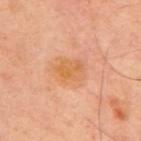Automated image analysis of the tile measured a footprint of about 6.5 mm², an eccentricity of roughly 0.7, and a shape-asymmetry score of about 0.2 (0 = symmetric). The software also gave a normalized lesion–skin contrast near 7. The analysis additionally found a nevus-likeness score of about 0/100 and a detector confidence of about 100 out of 100 that the crop contains a lesion. A roughly 15 mm field-of-view crop from a total-body skin photograph. On the back. Measured at roughly 3.5 mm in maximum diameter. The patient is a male in their 50s.